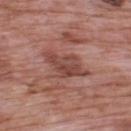Captured during whole-body skin photography for melanoma surveillance; the lesion was not biopsied.
A 15 mm close-up extracted from a 3D total-body photography capture.
From the upper back.
The total-body-photography lesion software estimated a lesion area of about 10 mm², an eccentricity of roughly 0.8, and a shape-asymmetry score of about 0.3 (0 = symmetric). The analysis additionally found roughly 9 lightness units darker than nearby skin. And it measured an automated nevus-likeness rating near 0 out of 100 and a lesion-detection confidence of about 100/100.
A male patient, in their 70s.
Imaged with white-light lighting.
Measured at roughly 4.5 mm in maximum diameter.